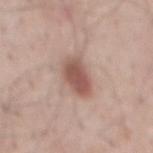Part of a total-body skin-imaging series; this lesion was reviewed on a skin check and was not flagged for biopsy. A region of skin cropped from a whole-body photographic capture, roughly 15 mm wide. Automated tile analysis of the lesion measured a nevus-likeness score of about 95/100 and lesion-presence confidence of about 100/100. A male patient about 40 years old. Captured under white-light illumination. On the back. Longest diameter approximately 5 mm.